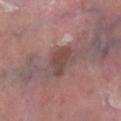Background: A close-up tile cropped from a whole-body skin photograph, about 15 mm across. From the right lower leg. The subject is a male approximately 40 years of age. The recorded lesion diameter is about 3.5 mm. This is a white-light tile.